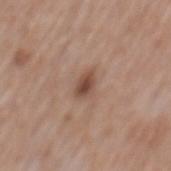Q: Was this lesion biopsied?
A: total-body-photography surveillance lesion; no biopsy
Q: Patient demographics?
A: male, in their 60s
Q: What is the imaging modality?
A: ~15 mm tile from a whole-body skin photo
Q: What did automated image analysis measure?
A: an area of roughly 3.5 mm², an eccentricity of roughly 0.75, and a symmetry-axis asymmetry near 0.2
Q: Where on the body is the lesion?
A: the back
Q: What lighting was used for the tile?
A: white-light
Q: How large is the lesion?
A: ~2.5 mm (longest diameter)